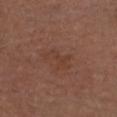Impression:
The lesion was photographed on a routine skin check and not biopsied; there is no pathology result.
Clinical summary:
The lesion is located on the head or neck. Captured under white-light illumination. A male subject, in their mid-70s. A lesion tile, about 15 mm wide, cut from a 3D total-body photograph.